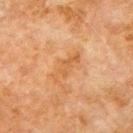<lesion>
  <patient>
    <sex>male</sex>
    <age_approx>80</age_approx>
  </patient>
  <image>
    <source>total-body photography crop</source>
    <field_of_view_mm>15</field_of_view_mm>
  </image>
  <site>left upper arm</site>
  <lighting>cross-polarized</lighting>
</lesion>